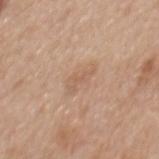The patient is a female in their 40s. The lesion is located on the mid back. Cropped from a whole-body photographic skin survey; the tile spans about 15 mm.